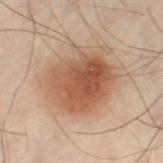This lesion was catalogued during total-body skin photography and was not selected for biopsy. This image is a 15 mm lesion crop taken from a total-body photograph. The lesion is on the left thigh. A male patient in their 50s.